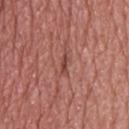Notes:
• biopsy status: total-body-photography surveillance lesion; no biopsy
• patient: male, roughly 75 years of age
• site: the upper back
• lighting: white-light
• automated lesion analysis: a footprint of about 2.5 mm² and two-axis asymmetry of about 0.35; a color-variation rating of about 0/10 and radial color variation of about 0; a classifier nevus-likeness of about 0/100 and a lesion-detection confidence of about 55/100
• image source: total-body-photography crop, ~15 mm field of view